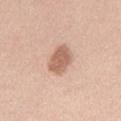subject: male, aged 38 to 42
lesion size: about 4 mm
lighting: white-light illumination
image: ~15 mm tile from a whole-body skin photo
location: the chest